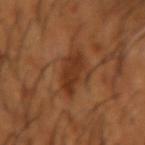workup: catalogued during a skin exam; not biopsied
anatomic site: the left forearm
image source: total-body-photography crop, ~15 mm field of view
patient: male, in their mid- to late 60s
tile lighting: cross-polarized illumination